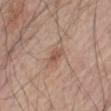Imaged during a routine full-body skin examination; the lesion was not biopsied and no histopathology is available. Automated image analysis of the tile measured an area of roughly 3 mm² and an outline eccentricity of about 0.8 (0 = round, 1 = elongated). The software also gave border irregularity of about 3 on a 0–10 scale, a within-lesion color-variation index near 1.5/10, and a peripheral color-asymmetry measure near 0.5. The lesion is located on the abdomen. This is a white-light tile. The lesion's longest dimension is about 2.5 mm. A 15 mm close-up tile from a total-body photography series done for melanoma screening. A male patient approximately 80 years of age.